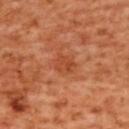<case>
<biopsy_status>not biopsied; imaged during a skin examination</biopsy_status>
<automated_metrics>
  <area_mm2_approx>4.5</area_mm2_approx>
  <eccentricity>0.65</eccentricity>
  <shape_asymmetry>0.25</shape_asymmetry>
  <cielab_L>49</cielab_L>
  <cielab_a>31</cielab_a>
  <cielab_b>39</cielab_b>
  <vs_skin_darker_L>7.0</vs_skin_darker_L>
  <vs_skin_contrast_norm>5.5</vs_skin_contrast_norm>
  <border_irregularity_0_10>2.0</border_irregularity_0_10>
  <peripheral_color_asymmetry>1.0</peripheral_color_asymmetry>
</automated_metrics>
<patient>
  <sex>female</sex>
  <age_approx>55</age_approx>
</patient>
<image>
  <source>total-body photography crop</source>
  <field_of_view_mm>15</field_of_view_mm>
</image>
<lesion_size>
  <long_diameter_mm_approx>2.5</long_diameter_mm_approx>
</lesion_size>
<lighting>cross-polarized</lighting>
<site>upper back</site>
</case>